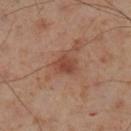The lesion was tiled from a total-body skin photograph and was not biopsied. Captured under cross-polarized illumination. A male subject approximately 55 years of age. A close-up tile cropped from a whole-body skin photograph, about 15 mm across. On the left lower leg. Longest diameter approximately 2.5 mm.